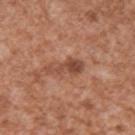No biopsy was performed on this lesion — it was imaged during a full skin examination and was not determined to be concerning. The lesion is on the arm. A male patient, in their mid-40s. Measured at roughly 4.5 mm in maximum diameter. A 15 mm close-up extracted from a 3D total-body photography capture.